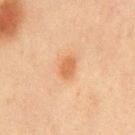Q: Is there a histopathology result?
A: imaged on a skin check; not biopsied
Q: What is the imaging modality?
A: ~15 mm tile from a whole-body skin photo
Q: What lighting was used for the tile?
A: cross-polarized
Q: Lesion location?
A: the chest
Q: What are the patient's age and sex?
A: male, aged around 45